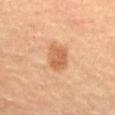Impression: Imaged during a routine full-body skin examination; the lesion was not biopsied and no histopathology is available. Acquisition and patient details: Approximately 3.5 mm at its widest. The tile uses cross-polarized illumination. The lesion is on the abdomen. The subject is a female aged approximately 60. A 15 mm crop from a total-body photograph taken for skin-cancer surveillance.